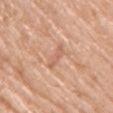| feature | finding |
|---|---|
| lighting | white-light illumination |
| patient | female, aged 63–67 |
| image source | ~15 mm tile from a whole-body skin photo |
| body site | the arm |
| lesion diameter | ≈3 mm |